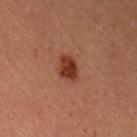Captured during whole-body skin photography for melanoma surveillance; the lesion was not biopsied. About 2.5 mm across. A female patient about 35 years old. The lesion is located on the left upper arm. Cropped from a whole-body photographic skin survey; the tile spans about 15 mm. Imaged with cross-polarized lighting.